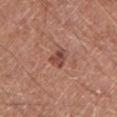Imaged during a routine full-body skin examination; the lesion was not biopsied and no histopathology is available.
A close-up tile cropped from a whole-body skin photograph, about 15 mm across.
Imaged with white-light lighting.
The subject is a male aged 78 to 82.
Located on the left lower leg.
About 2.5 mm across.